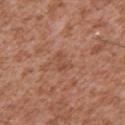Part of a total-body skin-imaging series; this lesion was reviewed on a skin check and was not flagged for biopsy. The subject is a male aged 43–47. The lesion-visualizer software estimated a footprint of about 3.5 mm², an eccentricity of roughly 0.75, and a shape-asymmetry score of about 0.5 (0 = symmetric). It also reported roughly 6 lightness units darker than nearby skin and a normalized border contrast of about 5. And it measured a nevus-likeness score of about 0/100. The lesion is located on the chest. A close-up tile cropped from a whole-body skin photograph, about 15 mm across. About 2.5 mm across.